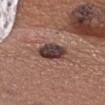| feature | finding |
|---|---|
| biopsy status | imaged on a skin check; not biopsied |
| body site | the head or neck |
| acquisition | 15 mm crop, total-body photography |
| patient | male, aged around 35 |